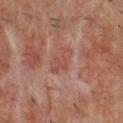notes: total-body-photography surveillance lesion; no biopsy
site: the chest
lighting: cross-polarized illumination
patient: male, about 50 years old
automated lesion analysis: an average lesion color of about L≈37 a*≈19 b*≈21 (CIELAB) and about 5 CIELAB-L* units darker than the surrounding skin
image source: ~15 mm tile from a whole-body skin photo
size: about 3.5 mm